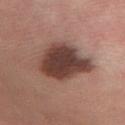Captured during whole-body skin photography for melanoma surveillance; the lesion was not biopsied. Located on the left forearm. Cropped from a whole-body photographic skin survey; the tile spans about 15 mm. A male subject, aged around 35.